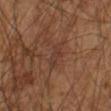Notes:
- notes · imaged on a skin check; not biopsied
- patient · male, about 65 years old
- image source · total-body-photography crop, ~15 mm field of view
- image-analysis metrics · a shape eccentricity near 0.85; a color-variation rating of about 1.5/10 and peripheral color asymmetry of about 0.5; an automated nevus-likeness rating near 0 out of 100 and a lesion-detection confidence of about 95/100
- location · the left arm
- diameter · ≈3 mm